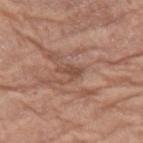Impression:
Recorded during total-body skin imaging; not selected for excision or biopsy.
Clinical summary:
Located on the leg. A region of skin cropped from a whole-body photographic capture, roughly 15 mm wide. Automated tile analysis of the lesion measured an area of roughly 2.5 mm² and an eccentricity of roughly 0.85. The software also gave a lesion color around L≈48 a*≈21 b*≈27 in CIELAB and about 9 CIELAB-L* units darker than the surrounding skin. The software also gave border irregularity of about 4 on a 0–10 scale. The analysis additionally found an automated nevus-likeness rating near 0 out of 100 and a lesion-detection confidence of about 75/100. The patient is a female aged approximately 75. The lesion's longest dimension is about 2.5 mm. Captured under white-light illumination.